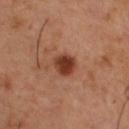follow-up = total-body-photography surveillance lesion; no biopsy
acquisition = ~15 mm tile from a whole-body skin photo
anatomic site = the upper back
patient = male, aged around 55
automated metrics = a border-irregularity index near 1.5/10 and a color-variation rating of about 3/10; an automated nevus-likeness rating near 95 out of 100 and a lesion-detection confidence of about 100/100
lighting = cross-polarized
lesion diameter = ~3 mm (longest diameter)